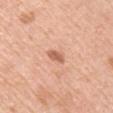notes: catalogued during a skin exam; not biopsied
tile lighting: white-light
subject: male, in their mid- to late 50s
anatomic site: the left upper arm
acquisition: ~15 mm crop, total-body skin-cancer survey